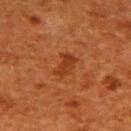A female patient approximately 50 years of age. The lesion-visualizer software estimated a mean CIELAB color near L≈31 a*≈26 b*≈33, about 7 CIELAB-L* units darker than the surrounding skin, and a normalized border contrast of about 6.5. The analysis additionally found a border-irregularity index near 3/10, internal color variation of about 2 on a 0–10 scale, and peripheral color asymmetry of about 0.5. The analysis additionally found an automated nevus-likeness rating near 20 out of 100. About 3 mm across. The lesion is located on the back. This is a cross-polarized tile. This image is a 15 mm lesion crop taken from a total-body photograph.